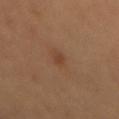Part of a total-body skin-imaging series; this lesion was reviewed on a skin check and was not flagged for biopsy.
A lesion tile, about 15 mm wide, cut from a 3D total-body photograph.
The tile uses cross-polarized illumination.
From the back.
Automated tile analysis of the lesion measured a mean CIELAB color near L≈40 a*≈21 b*≈30 and a normalized border contrast of about 6. It also reported border irregularity of about 2.5 on a 0–10 scale, a color-variation rating of about 0/10, and radial color variation of about 0.
Longest diameter approximately 2 mm.
A female patient.